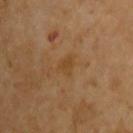{"biopsy_status": "not biopsied; imaged during a skin examination", "lighting": "cross-polarized", "image": {"source": "total-body photography crop", "field_of_view_mm": 15}, "patient": {"sex": "male", "age_approx": 65}, "site": "front of the torso"}